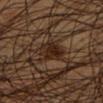{"biopsy_status": "not biopsied; imaged during a skin examination", "automated_metrics": {"area_mm2_approx": 7.0, "eccentricity": 0.15, "shape_asymmetry": 0.15, "nevus_likeness_0_100": 60, "lesion_detection_confidence_0_100": 95}, "lesion_size": {"long_diameter_mm_approx": 3.0}, "site": "left lower leg", "lighting": "cross-polarized", "patient": {"sex": "male", "age_approx": 45}, "image": {"source": "total-body photography crop", "field_of_view_mm": 15}}